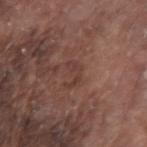<case>
<biopsy_status>not biopsied; imaged during a skin examination</biopsy_status>
<lesion_size>
  <long_diameter_mm_approx>2.5</long_diameter_mm_approx>
</lesion_size>
<lighting>white-light</lighting>
<site>left forearm</site>
<image>
  <source>total-body photography crop</source>
  <field_of_view_mm>15</field_of_view_mm>
</image>
<automated_metrics>
  <color_variation_0_10>0.0</color_variation_0_10>
  <peripheral_color_asymmetry>0.0</peripheral_color_asymmetry>
</automated_metrics>
<patient>
  <sex>male</sex>
  <age_approx>75</age_approx>
</patient>
</case>